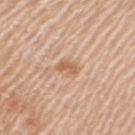An algorithmic analysis of the crop reported a shape eccentricity near 0.85. The subject is a female about 65 years old. Imaged with white-light lighting. A 15 mm close-up extracted from a 3D total-body photography capture. Located on the arm. The recorded lesion diameter is about 2.5 mm.